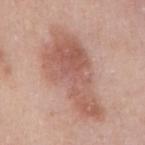The lesion was photographed on a routine skin check and not biopsied; there is no pathology result.
The subject is a female about 40 years old.
This image is a 15 mm lesion crop taken from a total-body photograph.
Located on the back.